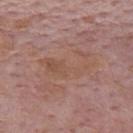Q: Was this lesion biopsied?
A: total-body-photography surveillance lesion; no biopsy
Q: Who is the patient?
A: male, aged 73–77
Q: How was this image acquired?
A: 15 mm crop, total-body photography
Q: How was the tile lit?
A: white-light illumination
Q: Lesion location?
A: the back
Q: Lesion size?
A: ~6 mm (longest diameter)